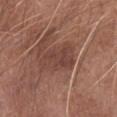Clinical impression: No biopsy was performed on this lesion — it was imaged during a full skin examination and was not determined to be concerning. Background: Imaged with white-light lighting. The recorded lesion diameter is about 5 mm. A male subject, aged 63 to 67. A lesion tile, about 15 mm wide, cut from a 3D total-body photograph. The lesion is located on the left forearm.